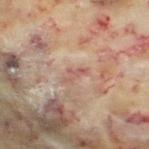{
  "biopsy_status": "not biopsied; imaged during a skin examination",
  "site": "left thigh",
  "lighting": "cross-polarized",
  "image": {
    "source": "total-body photography crop",
    "field_of_view_mm": 15
  },
  "patient": {
    "sex": "female",
    "age_approx": 60
  }
}